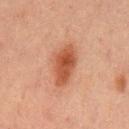notes = no biopsy performed (imaged during a skin exam)
size = ~5 mm (longest diameter)
site = the mid back
image source = total-body-photography crop, ~15 mm field of view
automated lesion analysis = a shape eccentricity near 0.85 and two-axis asymmetry of about 0.25; a border-irregularity index near 2.5/10, a color-variation rating of about 3.5/10, and radial color variation of about 1
subject = male, aged 63 to 67